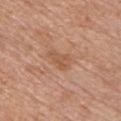biopsy status: total-body-photography surveillance lesion; no biopsy | body site: the chest | subject: male, approximately 60 years of age | image: ~15 mm crop, total-body skin-cancer survey.